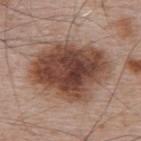Recorded during total-body skin imaging; not selected for excision or biopsy.
Approximately 8.5 mm at its widest.
The tile uses white-light illumination.
A male subject, aged 63 to 67.
The lesion is located on the upper back.
A close-up tile cropped from a whole-body skin photograph, about 15 mm across.
The total-body-photography lesion software estimated a footprint of about 43 mm², an outline eccentricity of about 0.65 (0 = round, 1 = elongated), and a symmetry-axis asymmetry near 0.2. The software also gave an average lesion color of about L≈44 a*≈20 b*≈26 (CIELAB), roughly 16 lightness units darker than nearby skin, and a normalized border contrast of about 12. The software also gave a border-irregularity index near 2.5/10, internal color variation of about 8 on a 0–10 scale, and peripheral color asymmetry of about 2.5.